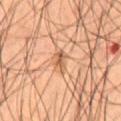Q: Is there a histopathology result?
A: no biopsy performed (imaged during a skin exam)
Q: Patient demographics?
A: male, aged 53 to 57
Q: Lesion location?
A: the front of the torso
Q: What lighting was used for the tile?
A: cross-polarized
Q: What is the lesion's diameter?
A: ≈2.5 mm
Q: What kind of image is this?
A: ~15 mm tile from a whole-body skin photo